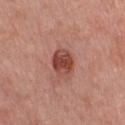This lesion was catalogued during total-body skin photography and was not selected for biopsy. Located on the chest. A roughly 15 mm field-of-view crop from a total-body skin photograph. The subject is a male aged approximately 60.